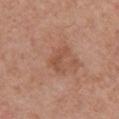Findings:
– biopsy status · total-body-photography surveillance lesion; no biopsy
– anatomic site · the chest
– subject · male, about 80 years old
– illumination · white-light illumination
– lesion diameter · ≈3.5 mm
– image · ~15 mm tile from a whole-body skin photo